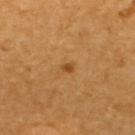Q: Was this lesion biopsied?
A: imaged on a skin check; not biopsied
Q: Where on the body is the lesion?
A: the upper back
Q: Illumination type?
A: cross-polarized illumination
Q: Automated lesion metrics?
A: an automated nevus-likeness rating near 80 out of 100 and a detector confidence of about 100 out of 100 that the crop contains a lesion
Q: How was this image acquired?
A: ~15 mm crop, total-body skin-cancer survey
Q: Patient demographics?
A: male, aged 48–52
Q: How large is the lesion?
A: about 1.5 mm